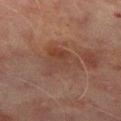Imaged during a routine full-body skin examination; the lesion was not biopsied and no histopathology is available. A roughly 15 mm field-of-view crop from a total-body skin photograph. A male subject, aged 68–72. On the right thigh.